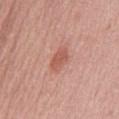Captured during whole-body skin photography for melanoma surveillance; the lesion was not biopsied. Approximately 3 mm at its widest. A male patient in their mid-70s. The tile uses white-light illumination. A 15 mm crop from a total-body photograph taken for skin-cancer surveillance. Located on the mid back. An algorithmic analysis of the crop reported a footprint of about 5 mm², an eccentricity of roughly 0.85, and a symmetry-axis asymmetry near 0.3. The analysis additionally found an average lesion color of about L≈56 a*≈27 b*≈29 (CIELAB), a lesion–skin lightness drop of about 9, and a normalized lesion–skin contrast near 6.5. The analysis additionally found a within-lesion color-variation index near 1.5/10 and radial color variation of about 0.5.